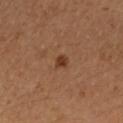Q: Is there a histopathology result?
A: catalogued during a skin exam; not biopsied
Q: How was the tile lit?
A: cross-polarized
Q: Where on the body is the lesion?
A: the arm
Q: Patient demographics?
A: female, aged 43–47
Q: Automated lesion metrics?
A: an area of roughly 2.5 mm² and an eccentricity of roughly 0.3; an automated nevus-likeness rating near 90 out of 100 and lesion-presence confidence of about 100/100
Q: What is the imaging modality?
A: ~15 mm crop, total-body skin-cancer survey
Q: Lesion size?
A: ≈1.5 mm